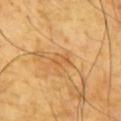Q: Was this lesion biopsied?
A: catalogued during a skin exam; not biopsied
Q: How large is the lesion?
A: ≈2.5 mm
Q: What is the anatomic site?
A: the chest
Q: What is the imaging modality?
A: 15 mm crop, total-body photography
Q: Illumination type?
A: cross-polarized
Q: Who is the patient?
A: male, roughly 65 years of age
Q: Automated lesion metrics?
A: a lesion area of about 3 mm² and two-axis asymmetry of about 0.3; a mean CIELAB color near L≈60 a*≈23 b*≈46, about 7 CIELAB-L* units darker than the surrounding skin, and a normalized lesion–skin contrast near 5